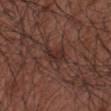– follow-up: imaged on a skin check; not biopsied
– subject: male, roughly 55 years of age
– lesion diameter: about 3 mm
– lighting: white-light
– image: total-body-photography crop, ~15 mm field of view
– automated metrics: a symmetry-axis asymmetry near 0.35; an average lesion color of about L≈30 a*≈19 b*≈21 (CIELAB) and a normalized border contrast of about 7; border irregularity of about 3.5 on a 0–10 scale, internal color variation of about 4.5 on a 0–10 scale, and radial color variation of about 1.5; an automated nevus-likeness rating near 5 out of 100 and lesion-presence confidence of about 95/100
– body site: the right forearm